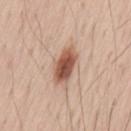Clinical impression: The lesion was photographed on a routine skin check and not biopsied; there is no pathology result. Image and clinical context: Cropped from a whole-body photographic skin survey; the tile spans about 15 mm. Located on the lower back. A male subject aged approximately 55.